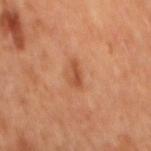This lesion was catalogued during total-body skin photography and was not selected for biopsy.